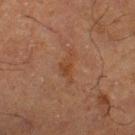Context: The lesion is located on the leg. Cropped from a whole-body photographic skin survey; the tile spans about 15 mm. A male patient aged 63 to 67. Automated image analysis of the tile measured an eccentricity of roughly 0.9 and two-axis asymmetry of about 0.55. It also reported an automated nevus-likeness rating near 0 out of 100. The recorded lesion diameter is about 4 mm. This is a cross-polarized tile.